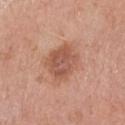Case summary:
- follow-up: imaged on a skin check; not biopsied
- image-analysis metrics: border irregularity of about 1.5 on a 0–10 scale and a color-variation rating of about 4/10
- lighting: white-light illumination
- lesion diameter: ≈4 mm
- patient: female, about 65 years old
- acquisition: ~15 mm crop, total-body skin-cancer survey
- site: the right forearm